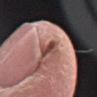Captured during whole-body skin photography for melanoma surveillance; the lesion was not biopsied.
On the right forearm.
Measured at roughly 2.5 mm in maximum diameter.
The patient is a female roughly 70 years of age.
A 15 mm crop from a total-body photograph taken for skin-cancer surveillance.
Imaged with white-light lighting.